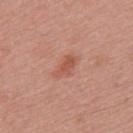{"biopsy_status": "not biopsied; imaged during a skin examination", "lighting": "white-light", "patient": {"sex": "male", "age_approx": 50}, "automated_metrics": {"area_mm2_approx": 4.5, "eccentricity": 0.9, "shape_asymmetry": 0.4, "color_variation_0_10": 1.5, "peripheral_color_asymmetry": 0.5}, "site": "upper back", "image": {"source": "total-body photography crop", "field_of_view_mm": 15}}